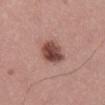Captured during whole-body skin photography for melanoma surveillance; the lesion was not biopsied. A roughly 15 mm field-of-view crop from a total-body skin photograph. The lesion is on the right thigh. A male patient, aged 53 to 57.